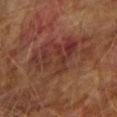Q: Is there a histopathology result?
A: total-body-photography surveillance lesion; no biopsy
Q: Patient demographics?
A: male, about 75 years old
Q: What is the anatomic site?
A: the right upper arm
Q: Lesion size?
A: ~8 mm (longest diameter)
Q: What lighting was used for the tile?
A: cross-polarized illumination
Q: What kind of image is this?
A: ~15 mm tile from a whole-body skin photo